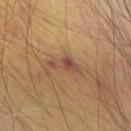Q: Was a biopsy performed?
A: catalogued during a skin exam; not biopsied
Q: How was this image acquired?
A: ~15 mm tile from a whole-body skin photo
Q: Where on the body is the lesion?
A: the back
Q: Who is the patient?
A: male, about 65 years old